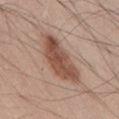Clinical impression: Part of a total-body skin-imaging series; this lesion was reviewed on a skin check and was not flagged for biopsy. Context: The patient is a male approximately 45 years of age. From the abdomen. A 15 mm close-up extracted from a 3D total-body photography capture.